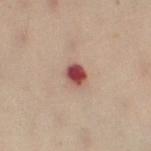No biopsy was performed on this lesion — it was imaged during a full skin examination and was not determined to be concerning.
A female subject, about 50 years old.
The lesion is located on the left thigh.
Cropped from a whole-body photographic skin survey; the tile spans about 15 mm.
This is a cross-polarized tile.
Measured at roughly 2.5 mm in maximum diameter.